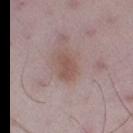biopsy_status: not biopsied; imaged during a skin examination
patient:
  sex: male
  age_approx: 50
image:
  source: total-body photography crop
  field_of_view_mm: 15
site: left thigh
lighting: white-light
lesion_size:
  long_diameter_mm_approx: 3.5